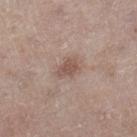Case summary:
– workup · total-body-photography surveillance lesion; no biopsy
– anatomic site · the right lower leg
– image source · ~15 mm crop, total-body skin-cancer survey
– patient · female, aged 58–62
– size · about 2.5 mm